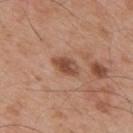Impression: Recorded during total-body skin imaging; not selected for excision or biopsy. Acquisition and patient details: The lesion is on the mid back. A male patient aged approximately 55. A region of skin cropped from a whole-body photographic capture, roughly 15 mm wide.